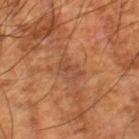This lesion was catalogued during total-body skin photography and was not selected for biopsy.
From the right lower leg.
Longest diameter approximately 3.5 mm.
A male subject, aged around 60.
An algorithmic analysis of the crop reported a lesion color around L≈47 a*≈24 b*≈33 in CIELAB, about 7 CIELAB-L* units darker than the surrounding skin, and a lesion-to-skin contrast of about 5.5 (normalized; higher = more distinct). The software also gave a border-irregularity index near 4/10, a color-variation rating of about 4/10, and a peripheral color-asymmetry measure near 1.5. And it measured a classifier nevus-likeness of about 0/100 and a detector confidence of about 95 out of 100 that the crop contains a lesion.
A 15 mm crop from a total-body photograph taken for skin-cancer surveillance.